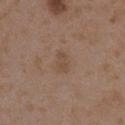Q: Was a biopsy performed?
A: catalogued during a skin exam; not biopsied
Q: What is the imaging modality?
A: ~15 mm tile from a whole-body skin photo
Q: Illumination type?
A: white-light
Q: Lesion location?
A: the right upper arm
Q: Patient demographics?
A: female, aged 33–37
Q: Automated lesion metrics?
A: internal color variation of about 1.5 on a 0–10 scale and radial color variation of about 0.5; a nevus-likeness score of about 0/100 and a lesion-detection confidence of about 100/100